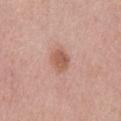biopsy_status: not biopsied; imaged during a skin examination
patient:
  sex: male
  age_approx: 70
lighting: white-light
image:
  source: total-body photography crop
  field_of_view_mm: 15
lesion_size:
  long_diameter_mm_approx: 3.0
site: mid back